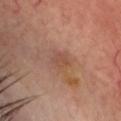size=≈2.5 mm | lighting=cross-polarized illumination | imaging modality=~15 mm crop, total-body skin-cancer survey | body site=the head or neck | patient=male, aged around 65.